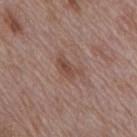workup = catalogued during a skin exam; not biopsied | site = the mid back | acquisition = total-body-photography crop, ~15 mm field of view | illumination = white-light | subject = male, approximately 70 years of age | diameter = ≈4 mm.